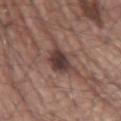Q: Is there a histopathology result?
A: catalogued during a skin exam; not biopsied
Q: What did automated image analysis measure?
A: an area of roughly 9.5 mm², a shape eccentricity near 0.5, and a symmetry-axis asymmetry near 0.35; a lesion color around L≈39 a*≈18 b*≈20 in CIELAB, about 11 CIELAB-L* units darker than the surrounding skin, and a normalized border contrast of about 9.5; a border-irregularity rating of about 4.5/10 and internal color variation of about 4 on a 0–10 scale
Q: What kind of image is this?
A: 15 mm crop, total-body photography
Q: What lighting was used for the tile?
A: white-light illumination
Q: How large is the lesion?
A: about 4 mm
Q: Lesion location?
A: the right upper arm
Q: Who is the patient?
A: male, in their mid-60s